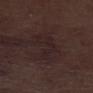<tbp_lesion>
  <biopsy_status>not biopsied; imaged during a skin examination</biopsy_status>
  <patient>
    <sex>male</sex>
    <age_approx>70</age_approx>
  </patient>
  <lighting>white-light</lighting>
  <image>
    <source>total-body photography crop</source>
    <field_of_view_mm>15</field_of_view_mm>
  </image>
  <site>left lower leg</site>
</tbp_lesion>